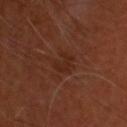biopsy_status: not biopsied; imaged during a skin examination